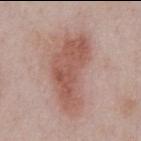This lesion was catalogued during total-body skin photography and was not selected for biopsy. This is a white-light tile. On the chest. A 15 mm close-up extracted from a 3D total-body photography capture. Measured at roughly 9 mm in maximum diameter. The patient is a male in their mid- to late 50s.